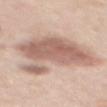Imaged during a routine full-body skin examination; the lesion was not biopsied and no histopathology is available. Captured under white-light illumination. The patient is a female roughly 65 years of age. Located on the mid back. A lesion tile, about 15 mm wide, cut from a 3D total-body photograph. The total-body-photography lesion software estimated a detector confidence of about 100 out of 100 that the crop contains a lesion. Longest diameter approximately 8 mm.